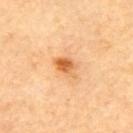Recorded during total-body skin imaging; not selected for excision or biopsy.
This image is a 15 mm lesion crop taken from a total-body photograph.
On the upper back.
A male subject, aged 53–57.
Measured at roughly 3.5 mm in maximum diameter.
Imaged with cross-polarized lighting.